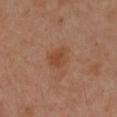Q: Is there a histopathology result?
A: imaged on a skin check; not biopsied
Q: What is the lesion's diameter?
A: ~3 mm (longest diameter)
Q: Who is the patient?
A: female, aged 38–42
Q: How was the tile lit?
A: cross-polarized illumination
Q: Lesion location?
A: the left arm
Q: How was this image acquired?
A: 15 mm crop, total-body photography